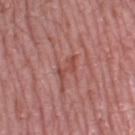| field | value |
|---|---|
| image source | 15 mm crop, total-body photography |
| patient | female, aged around 60 |
| anatomic site | the left thigh |